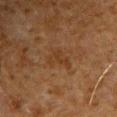biopsy_status: not biopsied; imaged during a skin examination
patient:
  sex: male
  age_approx: 80
lesion_size:
  long_diameter_mm_approx: 4.0
image:
  source: total-body photography crop
  field_of_view_mm: 15
site: left upper arm
automated_metrics:
  area_mm2_approx: 6.0
  eccentricity: 0.7
  shape_asymmetry: 0.5
  cielab_L: 29
  cielab_a: 16
  cielab_b: 27
  vs_skin_darker_L: 4.0
  vs_skin_contrast_norm: 5.0
  border_irregularity_0_10: 5.5
  color_variation_0_10: 1.5
  peripheral_color_asymmetry: 0.5
lighting: cross-polarized